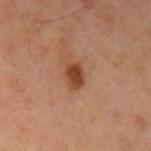Assessment:
Captured during whole-body skin photography for melanoma surveillance; the lesion was not biopsied.
Context:
The subject is a male roughly 40 years of age. A close-up tile cropped from a whole-body skin photograph, about 15 mm across. On the left upper arm.